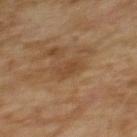<record>
  <biopsy_status>not biopsied; imaged during a skin examination</biopsy_status>
  <patient>
    <sex>female</sex>
    <age_approx>60</age_approx>
  </patient>
  <image>
    <source>total-body photography crop</source>
    <field_of_view_mm>15</field_of_view_mm>
  </image>
  <automated_metrics>
    <area_mm2_approx>5.0</area_mm2_approx>
    <eccentricity>0.7</eccentricity>
    <shape_asymmetry>0.25</shape_asymmetry>
    <cielab_L>38</cielab_L>
    <cielab_a>17</cielab_a>
    <cielab_b>30</cielab_b>
    <vs_skin_darker_L>6.0</vs_skin_darker_L>
    <vs_skin_contrast_norm>5.5</vs_skin_contrast_norm>
    <border_irregularity_0_10>2.5</border_irregularity_0_10>
    <color_variation_0_10>1.5</color_variation_0_10>
    <peripheral_color_asymmetry>0.5</peripheral_color_asymmetry>
  </automated_metrics>
  <lesion_size>
    <long_diameter_mm_approx>3.0</long_diameter_mm_approx>
  </lesion_size>
  <site>upper back</site>
  <lighting>cross-polarized</lighting>
</record>